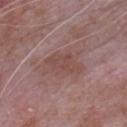{"biopsy_status": "not biopsied; imaged during a skin examination", "lighting": "white-light", "site": "front of the torso", "patient": {"sex": "male", "age_approx": 65}, "image": {"source": "total-body photography crop", "field_of_view_mm": 15}, "lesion_size": {"long_diameter_mm_approx": 4.0}}